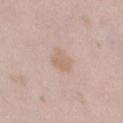Imaged during a routine full-body skin examination; the lesion was not biopsied and no histopathology is available.
Cropped from a whole-body photographic skin survey; the tile spans about 15 mm.
A female patient, roughly 25 years of age.
The lesion-visualizer software estimated an average lesion color of about L≈64 a*≈16 b*≈28 (CIELAB) and a lesion-to-skin contrast of about 5.5 (normalized; higher = more distinct). The software also gave internal color variation of about 1.5 on a 0–10 scale and a peripheral color-asymmetry measure near 0.5.
The tile uses white-light illumination.
On the left thigh.
The lesion's longest dimension is about 3 mm.